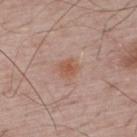Assessment: No biopsy was performed on this lesion — it was imaged during a full skin examination and was not determined to be concerning. Background: A male patient aged around 65. This is a white-light tile. Measured at roughly 2.5 mm in maximum diameter. The lesion is on the upper back. Automated image analysis of the tile measured a mean CIELAB color near L≈55 a*≈21 b*≈30 and a normalized lesion–skin contrast near 7. It also reported a color-variation rating of about 2.5/10 and peripheral color asymmetry of about 0.5. Cropped from a total-body skin-imaging series; the visible field is about 15 mm.